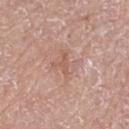| key | value |
|---|---|
| notes | catalogued during a skin exam; not biopsied |
| body site | the left thigh |
| image | total-body-photography crop, ~15 mm field of view |
| size | ≈2.5 mm |
| patient | female, aged 73 to 77 |
| tile lighting | white-light |